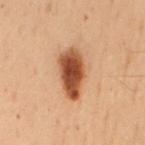| field | value |
|---|---|
| follow-up | imaged on a skin check; not biopsied |
| patient | female, aged 48 to 52 |
| acquisition | ~15 mm crop, total-body skin-cancer survey |
| location | the mid back |
| diameter | about 5.5 mm |